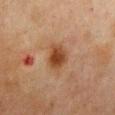biopsy status = imaged on a skin check; not biopsied
location = the chest
diameter = about 3.5 mm
TBP lesion metrics = an area of roughly 6.5 mm² and a symmetry-axis asymmetry near 0.2; a color-variation rating of about 3.5/10 and peripheral color asymmetry of about 1
subject = female, roughly 50 years of age
tile lighting = cross-polarized
image = total-body-photography crop, ~15 mm field of view